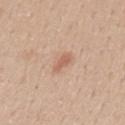| feature | finding |
|---|---|
| follow-up | total-body-photography surveillance lesion; no biopsy |
| imaging modality | 15 mm crop, total-body photography |
| image-analysis metrics | a footprint of about 3 mm², an outline eccentricity of about 0.9 (0 = round, 1 = elongated), and a shape-asymmetry score of about 0.3 (0 = symmetric) |
| lighting | white-light |
| location | the back |
| patient | male, aged 28 to 32 |
| lesion size | about 2.5 mm |